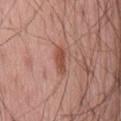<record>
  <biopsy_status>not biopsied; imaged during a skin examination</biopsy_status>
  <automated_metrics>
    <shape_asymmetry>0.2</shape_asymmetry>
    <cielab_L>50</cielab_L>
    <cielab_a>25</cielab_a>
    <cielab_b>29</cielab_b>
    <vs_skin_darker_L>10.0</vs_skin_darker_L>
    <vs_skin_contrast_norm>7.5</vs_skin_contrast_norm>
    <border_irregularity_0_10>2.5</border_irregularity_0_10>
    <color_variation_0_10>2.0</color_variation_0_10>
  </automated_metrics>
  <patient>
    <sex>male</sex>
    <age_approx>65</age_approx>
  </patient>
  <image>
    <source>total-body photography crop</source>
    <field_of_view_mm>15</field_of_view_mm>
  </image>
  <lesion_size>
    <long_diameter_mm_approx>3.5</long_diameter_mm_approx>
  </lesion_size>
  <lighting>white-light</lighting>
  <site>mid back</site>
</record>